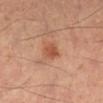Part of a total-body skin-imaging series; this lesion was reviewed on a skin check and was not flagged for biopsy. The lesion-visualizer software estimated a shape eccentricity near 0.65 and a symmetry-axis asymmetry near 0.2. It also reported an average lesion color of about L≈51 a*≈26 b*≈33 (CIELAB) and a normalized lesion–skin contrast near 7. The software also gave a border-irregularity rating of about 1.5/10, a color-variation rating of about 2/10, and peripheral color asymmetry of about 0.5. Captured under cross-polarized illumination. A male patient roughly 55 years of age. Approximately 2.5 mm at its widest. Cropped from a total-body skin-imaging series; the visible field is about 15 mm. Located on the right lower leg.